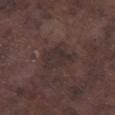No biopsy was performed on this lesion — it was imaged during a full skin examination and was not determined to be concerning. A 15 mm close-up extracted from a 3D total-body photography capture. Approximately 4.5 mm at its widest. A male patient in their mid- to late 70s. The total-body-photography lesion software estimated a lesion area of about 10 mm² and two-axis asymmetry of about 0.35. It also reported a border-irregularity rating of about 4/10 and a color-variation rating of about 2/10. It also reported a nevus-likeness score of about 0/100 and a detector confidence of about 85 out of 100 that the crop contains a lesion. The lesion is located on the right lower leg.